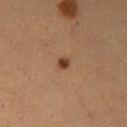Imaged during a routine full-body skin examination; the lesion was not biopsied and no histopathology is available.
The patient is a female in their mid-30s.
Automated tile analysis of the lesion measured an area of roughly 2.5 mm² and a shape-asymmetry score of about 0.2 (0 = symmetric). The software also gave a lesion color around L≈42 a*≈22 b*≈31 in CIELAB. And it measured a border-irregularity rating of about 1.5/10, a color-variation rating of about 1.5/10, and radial color variation of about 0.5. The analysis additionally found an automated nevus-likeness rating near 95 out of 100 and a lesion-detection confidence of about 100/100.
The lesion is located on the right upper arm.
Longest diameter approximately 1.5 mm.
Cropped from a whole-body photographic skin survey; the tile spans about 15 mm.
The tile uses cross-polarized illumination.